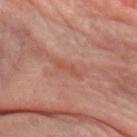| field | value |
|---|---|
| biopsy status | no biopsy performed (imaged during a skin exam) |
| patient | female, in their mid- to late 60s |
| anatomic site | the left forearm |
| TBP lesion metrics | a mean CIELAB color near L≈50 a*≈25 b*≈29, roughly 6 lightness units darker than nearby skin, and a lesion-to-skin contrast of about 5 (normalized; higher = more distinct); a border-irregularity index near 6/10 and a peripheral color-asymmetry measure near 0; a classifier nevus-likeness of about 0/100 |
| imaging modality | total-body-photography crop, ~15 mm field of view |
| illumination | cross-polarized illumination |